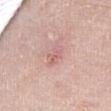notes — no biopsy performed (imaged during a skin exam)
patient — female, in their 70s
image — ~15 mm tile from a whole-body skin photo
lesion diameter — ~3 mm (longest diameter)
automated lesion analysis — an outline eccentricity of about 0.8 (0 = round, 1 = elongated) and a symmetry-axis asymmetry near 0.25; border irregularity of about 2.5 on a 0–10 scale and a within-lesion color-variation index near 5/10; an automated nevus-likeness rating near 0 out of 100 and lesion-presence confidence of about 100/100
location — the right thigh
tile lighting — white-light